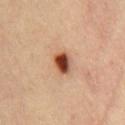The lesion was tiled from a total-body skin photograph and was not biopsied. The lesion is located on the chest. A region of skin cropped from a whole-body photographic capture, roughly 15 mm wide. A female subject aged 43–47.